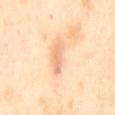Clinical summary:
A female patient about 55 years old. This is a cross-polarized tile. A 15 mm close-up tile from a total-body photography series done for melanoma screening. The lesion is located on the mid back.